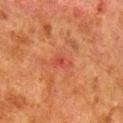image = total-body-photography crop, ~15 mm field of view | body site = the right lower leg | subject = male, aged around 80.